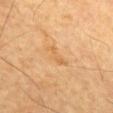Q: Is there a histopathology result?
A: no biopsy performed (imaged during a skin exam)
Q: What is the imaging modality?
A: total-body-photography crop, ~15 mm field of view
Q: Lesion location?
A: the front of the torso
Q: Who is the patient?
A: male, in their mid- to late 80s or older